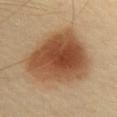Notes:
– notes · total-body-photography surveillance lesion; no biopsy
– patient · male, aged around 40
– imaging modality · total-body-photography crop, ~15 mm field of view
– site · the chest